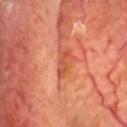| key | value |
|---|---|
| follow-up | catalogued during a skin exam; not biopsied |
| size | ≈3 mm |
| automated lesion analysis | a mean CIELAB color near L≈51 a*≈33 b*≈38, a lesion–skin lightness drop of about 9, and a normalized lesion–skin contrast near 6.5; lesion-presence confidence of about 80/100 |
| lighting | cross-polarized |
| image | total-body-photography crop, ~15 mm field of view |
| patient | male, approximately 65 years of age |
| site | the head or neck |